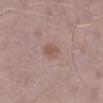  biopsy_status: not biopsied; imaged during a skin examination